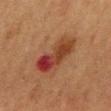Recorded during total-body skin imaging; not selected for excision or biopsy. Located on the mid back. A 15 mm close-up extracted from a 3D total-body photography capture. The subject is a male aged 73 to 77.